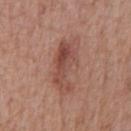| key | value |
|---|---|
| imaging modality | ~15 mm tile from a whole-body skin photo |
| lesion size | ≈6.5 mm |
| body site | the chest |
| subject | male, aged 63–67 |
| automated metrics | an eccentricity of roughly 0.9 |